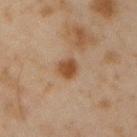This lesion was catalogued during total-body skin photography and was not selected for biopsy.